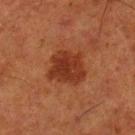Notes:
– workup — imaged on a skin check; not biopsied
– image — ~15 mm tile from a whole-body skin photo
– subject — male, aged around 80
– location — the left lower leg